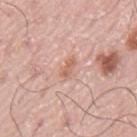workup: imaged on a skin check; not biopsied
lesion diameter: ≈2.5 mm
image: ~15 mm tile from a whole-body skin photo
tile lighting: white-light
image-analysis metrics: a footprint of about 3 mm² and an eccentricity of roughly 0.85; a mean CIELAB color near L≈63 a*≈23 b*≈29, roughly 9 lightness units darker than nearby skin, and a normalized lesion–skin contrast near 6; a lesion-detection confidence of about 100/100
subject: male, aged around 75
location: the mid back